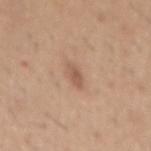The lesion was photographed on a routine skin check and not biopsied; there is no pathology result. About 2.5 mm across. A male subject, aged approximately 40. From the mid back. An algorithmic analysis of the crop reported an area of roughly 3 mm², an outline eccentricity of about 0.85 (0 = round, 1 = elongated), and a symmetry-axis asymmetry near 0.2. The analysis additionally found an average lesion color of about L≈56 a*≈20 b*≈29 (CIELAB) and roughly 9 lightness units darker than nearby skin. A 15 mm crop from a total-body photograph taken for skin-cancer surveillance. Captured under white-light illumination.